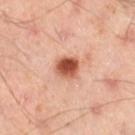Imaged with cross-polarized lighting.
A close-up tile cropped from a whole-body skin photograph, about 15 mm across.
The lesion-visualizer software estimated a border-irregularity rating of about 2/10 and a peripheral color-asymmetry measure near 2. It also reported a classifier nevus-likeness of about 100/100 and a detector confidence of about 100 out of 100 that the crop contains a lesion.
Longest diameter approximately 3 mm.
The lesion is on the left thigh.
A male patient, aged 43–47.